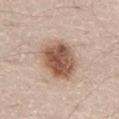biopsy_status: not biopsied; imaged during a skin examination
patient:
  sex: male
  age_approx: 80
image:
  source: total-body photography crop
  field_of_view_mm: 15
site: front of the torso